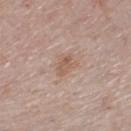illumination: white-light illumination; imaging modality: total-body-photography crop, ~15 mm field of view; patient: female, approximately 55 years of age; size: ~2.5 mm (longest diameter); site: the right lower leg.